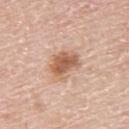Cropped from a whole-body photographic skin survey; the tile spans about 15 mm. A male patient about 60 years old. From the upper back.